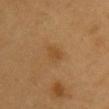biopsy status = catalogued during a skin exam; not biopsied | subject = male, in their 60s | acquisition = ~15 mm crop, total-body skin-cancer survey | anatomic site = the chest.